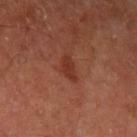<tbp_lesion>
<patient>
  <sex>male</sex>
  <age_approx>65</age_approx>
</patient>
<automated_metrics>
  <nevus_likeness_0_100>60</nevus_likeness_0_100>
  <lesion_detection_confidence_0_100>100</lesion_detection_confidence_0_100>
</automated_metrics>
<site>left upper arm</site>
<lesion_size>
  <long_diameter_mm_approx>3.0</long_diameter_mm_approx>
</lesion_size>
<image>
  <source>total-body photography crop</source>
  <field_of_view_mm>15</field_of_view_mm>
</image>
<lighting>cross-polarized</lighting>
</tbp_lesion>